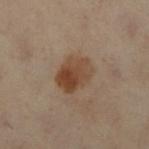biopsy status = catalogued during a skin exam; not biopsied | subject = female, aged 58 to 62 | site = the left leg | acquisition = total-body-photography crop, ~15 mm field of view | TBP lesion metrics = a lesion area of about 11 mm², an eccentricity of roughly 0.6, and two-axis asymmetry of about 0.15; a mean CIELAB color near L≈37 a*≈15 b*≈26, roughly 9 lightness units darker than nearby skin, and a normalized border contrast of about 9; a border-irregularity rating of about 1.5/10; a nevus-likeness score of about 100/100 | illumination = cross-polarized | lesion diameter = ~4 mm (longest diameter).